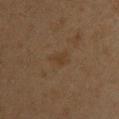Notes:
- subject: female, approximately 45 years of age
- image source: ~15 mm tile from a whole-body skin photo
- lighting: cross-polarized illumination
- lesion size: about 3 mm
- location: the upper back